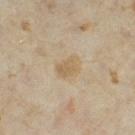follow-up: catalogued during a skin exam; not biopsied.